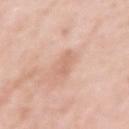Assessment:
This lesion was catalogued during total-body skin photography and was not selected for biopsy.
Background:
The subject is a female approximately 40 years of age. Measured at roughly 2.5 mm in maximum diameter. The tile uses white-light illumination. A 15 mm close-up extracted from a 3D total-body photography capture. Located on the left upper arm.